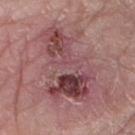patient = male, aged approximately 60
site = the arm
size = ~9 mm (longest diameter)
illumination = white-light
imaging modality = ~15 mm crop, total-body skin-cancer survey